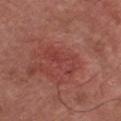The tile uses white-light illumination.
Automated tile analysis of the lesion measured a footprint of about 8.5 mm², a shape eccentricity near 0.9, and two-axis asymmetry of about 0.35. The analysis additionally found border irregularity of about 4.5 on a 0–10 scale, a color-variation rating of about 4/10, and a peripheral color-asymmetry measure near 1. The analysis additionally found a nevus-likeness score of about 25/100 and lesion-presence confidence of about 100/100.
A male subject roughly 75 years of age.
The lesion is located on the chest.
A lesion tile, about 15 mm wide, cut from a 3D total-body photograph.
Measured at roughly 5 mm in maximum diameter.